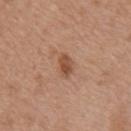{"biopsy_status": "not biopsied; imaged during a skin examination", "automated_metrics": {"area_mm2_approx": 4.0, "shape_asymmetry": 0.25, "cielab_L": 50, "cielab_a": 22, "cielab_b": 32, "vs_skin_darker_L": 10.0, "border_irregularity_0_10": 2.0}, "lighting": "white-light", "lesion_size": {"long_diameter_mm_approx": 3.0}, "patient": {"sex": "female", "age_approx": 75}, "image": {"source": "total-body photography crop", "field_of_view_mm": 15}, "site": "chest"}